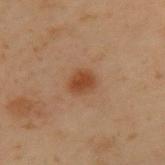<record>
<biopsy_status>not biopsied; imaged during a skin examination</biopsy_status>
<lesion_size>
  <long_diameter_mm_approx>2.5</long_diameter_mm_approx>
</lesion_size>
<patient>
  <sex>male</sex>
  <age_approx>55</age_approx>
</patient>
<site>upper back</site>
<image>
  <source>total-body photography crop</source>
  <field_of_view_mm>15</field_of_view_mm>
</image>
</record>